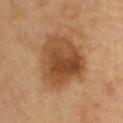Clinical impression:
Recorded during total-body skin imaging; not selected for excision or biopsy.
Acquisition and patient details:
A female patient aged approximately 45. From the upper back. A roughly 15 mm field-of-view crop from a total-body skin photograph. About 7 mm across. Captured under cross-polarized illumination. Automated tile analysis of the lesion measured a lesion–skin lightness drop of about 12. It also reported a border-irregularity rating of about 2.5/10.